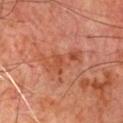workup: no biopsy performed (imaged during a skin exam) | tile lighting: cross-polarized illumination | subject: male, approximately 65 years of age | image-analysis metrics: a mean CIELAB color near L≈45 a*≈26 b*≈32, about 6 CIELAB-L* units darker than the surrounding skin, and a normalized border contrast of about 6; a border-irregularity rating of about 6.5/10, internal color variation of about 3 on a 0–10 scale, and peripheral color asymmetry of about 1 | anatomic site: the chest | size: about 5.5 mm | image: ~15 mm crop, total-body skin-cancer survey.